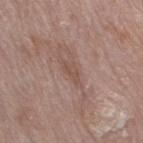No biopsy was performed on this lesion — it was imaged during a full skin examination and was not determined to be concerning. Cropped from a total-body skin-imaging series; the visible field is about 15 mm. Measured at roughly 2.5 mm in maximum diameter. On the right thigh. A female subject, roughly 70 years of age. The tile uses white-light illumination.